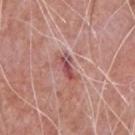notes: total-body-photography surveillance lesion; no biopsy | lesion size: ~3.5 mm (longest diameter) | body site: the chest | image source: total-body-photography crop, ~15 mm field of view | subject: male, aged 63–67 | automated lesion analysis: a lesion color around L≈51 a*≈28 b*≈22 in CIELAB, a lesion–skin lightness drop of about 11, and a lesion-to-skin contrast of about 7.5 (normalized; higher = more distinct); a border-irregularity index near 5/10, a color-variation rating of about 7.5/10, and radial color variation of about 2.5; a nevus-likeness score of about 0/100 and lesion-presence confidence of about 90/100.